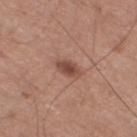The lesion was tiled from a total-body skin photograph and was not biopsied.
The lesion is on the left lower leg.
Approximately 3 mm at its widest.
This image is a 15 mm lesion crop taken from a total-body photograph.
A male patient, roughly 50 years of age.
Automated image analysis of the tile measured a lesion area of about 4.5 mm², a shape eccentricity near 0.75, and a symmetry-axis asymmetry near 0.25. The software also gave a lesion color around L≈48 a*≈22 b*≈26 in CIELAB, a lesion–skin lightness drop of about 11, and a normalized border contrast of about 8. And it measured a nevus-likeness score of about 90/100 and a detector confidence of about 100 out of 100 that the crop contains a lesion.
This is a white-light tile.